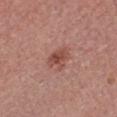Clinical impression:
The lesion was tiled from a total-body skin photograph and was not biopsied.
Context:
A female patient, aged approximately 25. The lesion is on the front of the torso. Cropped from a whole-body photographic skin survey; the tile spans about 15 mm. Imaged with white-light lighting. Automated tile analysis of the lesion measured a border-irregularity index near 3.5/10, internal color variation of about 4 on a 0–10 scale, and peripheral color asymmetry of about 1. Longest diameter approximately 3.5 mm.